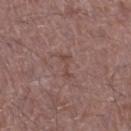biopsy_status: not biopsied; imaged during a skin examination
image:
  source: total-body photography crop
  field_of_view_mm: 15
lesion_size:
  long_diameter_mm_approx: 3.0
site: left thigh
patient:
  sex: male
  age_approx: 50
automated_metrics:
  area_mm2_approx: 3.0
  eccentricity: 0.9
  shape_asymmetry: 0.5